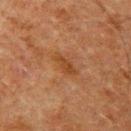{
  "biopsy_status": "not biopsied; imaged during a skin examination",
  "site": "left upper arm",
  "patient": {
    "sex": "male",
    "age_approx": 60
  },
  "lighting": "cross-polarized",
  "image": {
    "source": "total-body photography crop",
    "field_of_view_mm": 15
  },
  "lesion_size": {
    "long_diameter_mm_approx": 3.5
  }
}